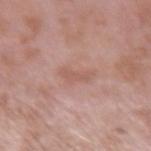- workup — imaged on a skin check; not biopsied
- patient — male, roughly 55 years of age
- body site — the left upper arm
- lesion size — ~3 mm (longest diameter)
- image — 15 mm crop, total-body photography